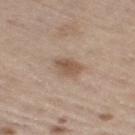Notes:
- biopsy status · catalogued during a skin exam; not biopsied
- imaging modality · total-body-photography crop, ~15 mm field of view
- subject · male, roughly 70 years of age
- site · the right thigh
- tile lighting · white-light illumination